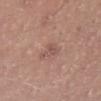No biopsy was performed on this lesion — it was imaged during a full skin examination and was not determined to be concerning. The tile uses white-light illumination. Located on the left lower leg. A 15 mm close-up extracted from a 3D total-body photography capture. The patient is a male about 40 years old. Approximately 3 mm at its widest.